Located on the arm; cropped from a total-body skin-imaging series; the visible field is about 15 mm; the patient is a male about 55 years old — 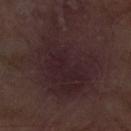About 9.5 mm across. This is a white-light tile. The biopsy diagnosis was a squamous cell carcinoma in situ, classified as a malignant lesion.